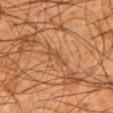| key | value |
|---|---|
| location | the left upper arm |
| TBP lesion metrics | a lesion area of about 2.5 mm², an outline eccentricity of about 0.85 (0 = round, 1 = elongated), and two-axis asymmetry of about 0.45; an average lesion color of about L≈48 a*≈22 b*≈35 (CIELAB), roughly 7 lightness units darker than nearby skin, and a normalized border contrast of about 5.5 |
| illumination | cross-polarized |
| size | ≈2.5 mm |
| image | 15 mm crop, total-body photography |
| subject | male, aged 43–47 |